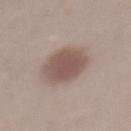The lesion was tiled from a total-body skin photograph and was not biopsied.
Approximately 5 mm at its widest.
The tile uses white-light illumination.
A roughly 15 mm field-of-view crop from a total-body skin photograph.
From the mid back.
A male subject, about 30 years old.